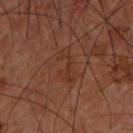Q: Is there a histopathology result?
A: imaged on a skin check; not biopsied
Q: How was this image acquired?
A: ~15 mm crop, total-body skin-cancer survey
Q: Lesion location?
A: the upper back
Q: Patient demographics?
A: male, about 60 years old